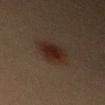<tbp_lesion>
  <biopsy_status>not biopsied; imaged during a skin examination</biopsy_status>
  <site>right upper arm</site>
  <lesion_size>
    <long_diameter_mm_approx>4.0</long_diameter_mm_approx>
  </lesion_size>
  <patient>
    <sex>male</sex>
    <age_approx>40</age_approx>
  </patient>
  <image>
    <source>total-body photography crop</source>
    <field_of_view_mm>15</field_of_view_mm>
  </image>
</tbp_lesion>